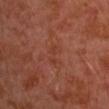Recorded during total-body skin imaging; not selected for excision or biopsy. Automated tile analysis of the lesion measured a lesion area of about 3.5 mm², an outline eccentricity of about 0.85 (0 = round, 1 = elongated), and a symmetry-axis asymmetry near 0.3. The software also gave a border-irregularity index near 4.5/10, a color-variation rating of about 1/10, and radial color variation of about 0.5. The analysis additionally found a nevus-likeness score of about 0/100 and a lesion-detection confidence of about 100/100. Longest diameter approximately 2.5 mm. The lesion is on the left arm. The tile uses cross-polarized illumination. A lesion tile, about 15 mm wide, cut from a 3D total-body photograph. A male subject, aged 28 to 32.